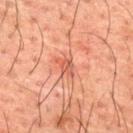Recorded during total-body skin imaging; not selected for excision or biopsy. Cropped from a total-body skin-imaging series; the visible field is about 15 mm. The lesion is on the mid back. This is a cross-polarized tile. Approximately 2.5 mm at its widest. The patient is a male aged 48–52.